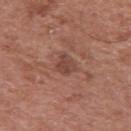Clinical impression: No biopsy was performed on this lesion — it was imaged during a full skin examination and was not determined to be concerning. Clinical summary: A roughly 15 mm field-of-view crop from a total-body skin photograph. A male subject aged 63–67. On the right upper arm.